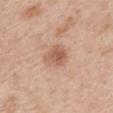Imaged during a routine full-body skin examination; the lesion was not biopsied and no histopathology is available.
Automated image analysis of the tile measured an average lesion color of about L≈58 a*≈21 b*≈31 (CIELAB), about 10 CIELAB-L* units darker than the surrounding skin, and a normalized lesion–skin contrast near 7. It also reported a nevus-likeness score of about 45/100 and a detector confidence of about 100 out of 100 that the crop contains a lesion.
A female subject, aged around 40.
The recorded lesion diameter is about 3 mm.
From the mid back.
This is a white-light tile.
A 15 mm close-up extracted from a 3D total-body photography capture.